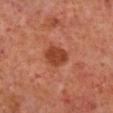This lesion was catalogued during total-body skin photography and was not selected for biopsy.
Longest diameter approximately 3 mm.
This is a cross-polarized tile.
Automated image analysis of the tile measured an area of roughly 6.5 mm², an outline eccentricity of about 0.6 (0 = round, 1 = elongated), and a symmetry-axis asymmetry near 0.25. And it measured a classifier nevus-likeness of about 85/100.
A male subject aged approximately 60.
A 15 mm close-up tile from a total-body photography series done for melanoma screening.
On the leg.